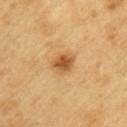Q: Was a biopsy performed?
A: no biopsy performed (imaged during a skin exam)
Q: Illumination type?
A: cross-polarized illumination
Q: What did automated image analysis measure?
A: a mean CIELAB color near L≈55 a*≈21 b*≈42 and about 13 CIELAB-L* units darker than the surrounding skin; a border-irregularity index near 2.5/10 and a within-lesion color-variation index near 3.5/10
Q: How large is the lesion?
A: ~3 mm (longest diameter)
Q: What kind of image is this?
A: total-body-photography crop, ~15 mm field of view
Q: What is the anatomic site?
A: the left upper arm
Q: Patient demographics?
A: male, in their 60s